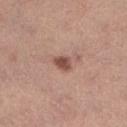Context:
About 2.5 mm across. Located on the right lower leg. The subject is a female approximately 65 years of age. Cropped from a total-body skin-imaging series; the visible field is about 15 mm.